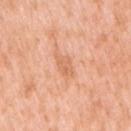Notes:
– follow-up: no biopsy performed (imaged during a skin exam)
– automated lesion analysis: an average lesion color of about L≈66 a*≈26 b*≈37 (CIELAB), a lesion–skin lightness drop of about 8, and a normalized border contrast of about 5; a border-irregularity rating of about 3/10, internal color variation of about 2.5 on a 0–10 scale, and radial color variation of about 1; a nevus-likeness score of about 0/100
– size: ~3 mm (longest diameter)
– image source: total-body-photography crop, ~15 mm field of view
– body site: the arm
– patient: female, aged 38 to 42
– tile lighting: white-light illumination